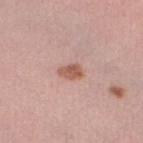| field | value |
|---|---|
| workup | imaged on a skin check; not biopsied |